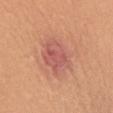The lesion was photographed on a routine skin check and not biopsied; there is no pathology result. Imaged with white-light lighting. A roughly 15 mm field-of-view crop from a total-body skin photograph. From the back. The subject is a female in their 40s. The recorded lesion diameter is about 5.5 mm. The total-body-photography lesion software estimated an average lesion color of about L≈57 a*≈28 b*≈28 (CIELAB), a lesion–skin lightness drop of about 9, and a lesion-to-skin contrast of about 7 (normalized; higher = more distinct). And it measured a border-irregularity rating of about 2.5/10, internal color variation of about 4 on a 0–10 scale, and radial color variation of about 1.5. And it measured an automated nevus-likeness rating near 30 out of 100 and a lesion-detection confidence of about 100/100.